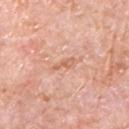patient = male, aged around 80 | lesion diameter = about 3 mm | image source = ~15 mm crop, total-body skin-cancer survey | tile lighting = white-light | anatomic site = the arm.